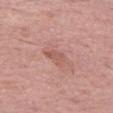Impression:
Part of a total-body skin-imaging series; this lesion was reviewed on a skin check and was not flagged for biopsy.
Background:
The recorded lesion diameter is about 2.5 mm. Cropped from a whole-body photographic skin survey; the tile spans about 15 mm. A female patient, approximately 60 years of age. On the left thigh. This is a white-light tile.